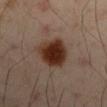Findings:
* body site: the left upper arm
* subject: male, aged 48 to 52
* image source: ~15 mm tile from a whole-body skin photo
* lighting: cross-polarized